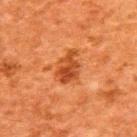Impression: Recorded during total-body skin imaging; not selected for excision or biopsy. Image and clinical context: The lesion-visualizer software estimated a footprint of about 8 mm², a shape eccentricity near 0.7, and two-axis asymmetry of about 0.25. And it measured an average lesion color of about L≈38 a*≈27 b*≈37 (CIELAB), a lesion–skin lightness drop of about 10, and a lesion-to-skin contrast of about 8 (normalized; higher = more distinct). It also reported a border-irregularity index near 3/10 and a peripheral color-asymmetry measure near 1.5. A male subject aged around 60. The recorded lesion diameter is about 4 mm. On the back. The tile uses cross-polarized illumination. Cropped from a whole-body photographic skin survey; the tile spans about 15 mm.